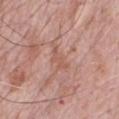notes: catalogued during a skin exam; not biopsied
image: total-body-photography crop, ~15 mm field of view
patient: male, approximately 70 years of age
illumination: white-light illumination
anatomic site: the chest